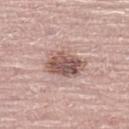Case summary:
• workup — imaged on a skin check; not biopsied
• patient — male, aged 68–72
• image-analysis metrics — a lesion area of about 11 mm² and a shape eccentricity near 0.75; a border-irregularity rating of about 2/10
• location — the leg
• image source — ~15 mm crop, total-body skin-cancer survey
• illumination — white-light illumination
• lesion size — ≈4.5 mm